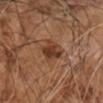Q: Was a biopsy performed?
A: total-body-photography surveillance lesion; no biopsy
Q: What kind of image is this?
A: 15 mm crop, total-body photography
Q: How was the tile lit?
A: cross-polarized illumination
Q: Lesion size?
A: ≈3.5 mm
Q: Patient demographics?
A: male, in their 60s
Q: Lesion location?
A: the right arm
Q: Automated lesion metrics?
A: a lesion area of about 6.5 mm²; a color-variation rating of about 4/10 and radial color variation of about 1.5; a classifier nevus-likeness of about 75/100 and lesion-presence confidence of about 100/100